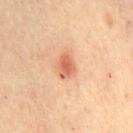Impression: Imaged during a routine full-body skin examination; the lesion was not biopsied and no histopathology is available. Background: A region of skin cropped from a whole-body photographic capture, roughly 15 mm wide. From the chest. The subject is a female aged 43–47. The total-body-photography lesion software estimated a border-irregularity index near 2/10, a within-lesion color-variation index near 3/10, and radial color variation of about 1. About 3 mm across. The tile uses cross-polarized illumination.